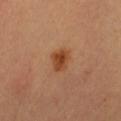| feature | finding |
|---|---|
| workup | total-body-photography surveillance lesion; no biopsy |
| subject | female, approximately 45 years of age |
| imaging modality | 15 mm crop, total-body photography |
| lesion size | ~3 mm (longest diameter) |
| illumination | cross-polarized illumination |
| body site | the abdomen |